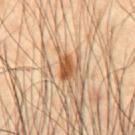Impression:
Recorded during total-body skin imaging; not selected for excision or biopsy.